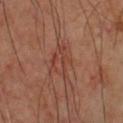Assessment:
No biopsy was performed on this lesion — it was imaged during a full skin examination and was not determined to be concerning.
Clinical summary:
The lesion is on the chest. Imaged with cross-polarized lighting. Cropped from a total-body skin-imaging series; the visible field is about 15 mm. The recorded lesion diameter is about 4 mm. A male patient, aged 58–62.